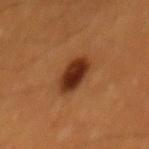No biopsy was performed on this lesion — it was imaged during a full skin examination and was not determined to be concerning.